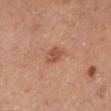notes = catalogued during a skin exam; not biopsied
site = the left lower leg
tile lighting = cross-polarized illumination
subject = male, aged approximately 60
image = total-body-photography crop, ~15 mm field of view
lesion diameter = about 2.5 mm